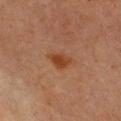follow-up=total-body-photography surveillance lesion; no biopsy.